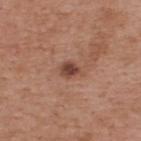A male patient, aged approximately 55. From the back. About 2.5 mm across. Cropped from a total-body skin-imaging series; the visible field is about 15 mm. This is a white-light tile.